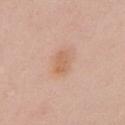Q: Was a biopsy performed?
A: imaged on a skin check; not biopsied
Q: What kind of image is this?
A: ~15 mm tile from a whole-body skin photo
Q: How large is the lesion?
A: ~3 mm (longest diameter)
Q: Who is the patient?
A: female, aged around 25
Q: Illumination type?
A: white-light illumination
Q: Where on the body is the lesion?
A: the left upper arm